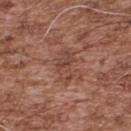{"biopsy_status": "not biopsied; imaged during a skin examination", "lighting": "white-light", "site": "upper back", "image": {"source": "total-body photography crop", "field_of_view_mm": 15}, "patient": {"sex": "male", "age_approx": 55}, "lesion_size": {"long_diameter_mm_approx": 4.0}}